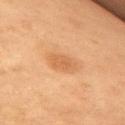<case>
<biopsy_status>not biopsied; imaged during a skin examination</biopsy_status>
<image>
  <source>total-body photography crop</source>
  <field_of_view_mm>15</field_of_view_mm>
</image>
<site>right upper arm</site>
<lesion_size>
  <long_diameter_mm_approx>3.5</long_diameter_mm_approx>
</lesion_size>
<patient>
  <sex>female</sex>
  <age_approx>60</age_approx>
</patient>
<lighting>cross-polarized</lighting>
</case>